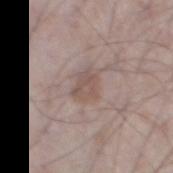Clinical impression:
The lesion was photographed on a routine skin check and not biopsied; there is no pathology result.
Clinical summary:
The tile uses white-light illumination. A male patient in their mid-60s. Longest diameter approximately 3 mm. On the left thigh. This image is a 15 mm lesion crop taken from a total-body photograph.